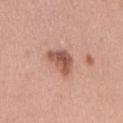  automated_metrics:
    area_mm2_approx: 8.0
    eccentricity: 0.55
    shape_asymmetry: 0.35
    vs_skin_contrast_norm: 8.5
  lesion_size:
    long_diameter_mm_approx: 3.5
  image:
    source: total-body photography crop
    field_of_view_mm: 15
  patient:
    sex: female
    age_approx: 35
  site: arm